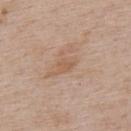biopsy status: no biopsy performed (imaged during a skin exam)
illumination: white-light illumination
subject: male, aged around 75
acquisition: ~15 mm tile from a whole-body skin photo
body site: the upper back
automated metrics: a lesion color around L≈57 a*≈19 b*≈31 in CIELAB and a normalized border contrast of about 5; a border-irregularity index near 3/10 and a color-variation rating of about 1.5/10; lesion-presence confidence of about 100/100
diameter: ~3 mm (longest diameter)